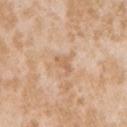Impression: Part of a total-body skin-imaging series; this lesion was reviewed on a skin check and was not flagged for biopsy. Context: The lesion is located on the right upper arm. Automated image analysis of the tile measured a lesion color around L≈63 a*≈19 b*≈35 in CIELAB and about 7 CIELAB-L* units darker than the surrounding skin. The analysis additionally found border irregularity of about 5 on a 0–10 scale and internal color variation of about 1 on a 0–10 scale. It also reported a classifier nevus-likeness of about 0/100 and a detector confidence of about 100 out of 100 that the crop contains a lesion. Measured at roughly 2.5 mm in maximum diameter. Cropped from a whole-body photographic skin survey; the tile spans about 15 mm. The tile uses white-light illumination. A female subject aged 23–27.